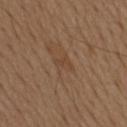Assessment:
Part of a total-body skin-imaging series; this lesion was reviewed on a skin check and was not flagged for biopsy.
Image and clinical context:
This is a white-light tile. A male patient approximately 75 years of age. From the mid back. The lesion's longest dimension is about 2.5 mm. Cropped from a whole-body photographic skin survey; the tile spans about 15 mm.